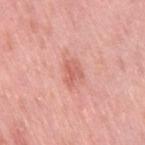The lesion was tiled from a total-body skin photograph and was not biopsied.
A close-up tile cropped from a whole-body skin photograph, about 15 mm across.
The lesion is on the right thigh.
Imaged with white-light lighting.
A female subject aged approximately 40.
Approximately 3 mm at its widest.
The total-body-photography lesion software estimated border irregularity of about 3.5 on a 0–10 scale, internal color variation of about 2.5 on a 0–10 scale, and radial color variation of about 1.